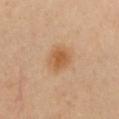Clinical impression: Recorded during total-body skin imaging; not selected for excision or biopsy. Context: On the chest. The subject is a female aged 38–42. A 15 mm close-up extracted from a 3D total-body photography capture.